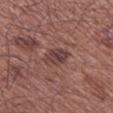Assessment: This lesion was catalogued during total-body skin photography and was not selected for biopsy. Context: Automated tile analysis of the lesion measured a mean CIELAB color near L≈40 a*≈20 b*≈20, a lesion–skin lightness drop of about 9, and a lesion-to-skin contrast of about 8 (normalized; higher = more distinct). The tile uses white-light illumination. Cropped from a whole-body photographic skin survey; the tile spans about 15 mm. The lesion is on the right lower leg. Approximately 3 mm at its widest. A male patient aged 63–67.